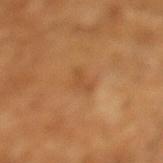Q: Automated lesion metrics?
A: a footprint of about 3.5 mm², an eccentricity of roughly 0.9, and a symmetry-axis asymmetry near 0.45; a border-irregularity index near 4.5/10 and radial color variation of about 0
Q: What is the imaging modality?
A: ~15 mm tile from a whole-body skin photo
Q: What are the patient's age and sex?
A: male, about 65 years old